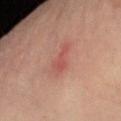workup = catalogued during a skin exam; not biopsied
imaging modality = ~15 mm crop, total-body skin-cancer survey
site = the left forearm
patient = male, approximately 65 years of age